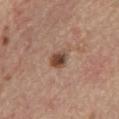Clinical impression:
This lesion was catalogued during total-body skin photography and was not selected for biopsy.
Background:
From the mid back. Automated image analysis of the tile measured a shape-asymmetry score of about 0.35 (0 = symmetric). It also reported an average lesion color of about L≈46 a*≈19 b*≈28 (CIELAB), about 13 CIELAB-L* units darker than the surrounding skin, and a normalized border contrast of about 9.5. And it measured a border-irregularity rating of about 3/10, a within-lesion color-variation index near 4.5/10, and radial color variation of about 1.5. It also reported an automated nevus-likeness rating near 95 out of 100 and a lesion-detection confidence of about 100/100. Approximately 3 mm at its widest. The patient is a male approximately 70 years of age. Captured under white-light illumination. Cropped from a whole-body photographic skin survey; the tile spans about 15 mm.